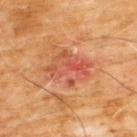This lesion was catalogued during total-body skin photography and was not selected for biopsy. About 5 mm across. From the chest. A 15 mm crop from a total-body photograph taken for skin-cancer surveillance. A male subject, about 60 years old.